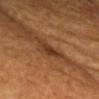Part of a total-body skin-imaging series; this lesion was reviewed on a skin check and was not flagged for biopsy. This image is a 15 mm lesion crop taken from a total-body photograph. Measured at roughly 4 mm in maximum diameter. From the chest. A female subject in their 70s. Automated image analysis of the tile measured a lesion color around L≈35 a*≈22 b*≈31 in CIELAB and about 10 CIELAB-L* units darker than the surrounding skin. The software also gave border irregularity of about 4 on a 0–10 scale, internal color variation of about 0.5 on a 0–10 scale, and peripheral color asymmetry of about 0.